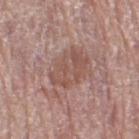Impression:
Part of a total-body skin-imaging series; this lesion was reviewed on a skin check and was not flagged for biopsy.
Acquisition and patient details:
Captured under white-light illumination. Cropped from a whole-body photographic skin survey; the tile spans about 15 mm. The subject is a female aged around 65. From the right thigh. Measured at roughly 5 mm in maximum diameter.